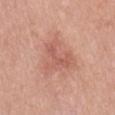* workup: catalogued during a skin exam; not biopsied
* lesion diameter: ≈6 mm
* lighting: white-light illumination
* subject: female, about 40 years old
* image: total-body-photography crop, ~15 mm field of view
* image-analysis metrics: a lesion area of about 17 mm² and an outline eccentricity of about 0.45 (0 = round, 1 = elongated); a color-variation rating of about 4/10; a nevus-likeness score of about 10/100 and a lesion-detection confidence of about 100/100
* location: the mid back